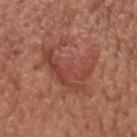{
  "biopsy_status": "not biopsied; imaged during a skin examination",
  "lighting": "white-light",
  "image": {
    "source": "total-body photography crop",
    "field_of_view_mm": 15
  },
  "site": "head or neck",
  "automated_metrics": {
    "cielab_L": 45,
    "cielab_a": 25,
    "cielab_b": 28,
    "vs_skin_darker_L": 7.0,
    "vs_skin_contrast_norm": 5.5,
    "nevus_likeness_0_100": 60,
    "lesion_detection_confidence_0_100": 100
  },
  "patient": {
    "sex": "male",
    "age_approx": 65
  },
  "lesion_size": {
    "long_diameter_mm_approx": 6.5
  }
}